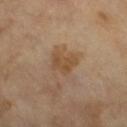follow-up — total-body-photography surveillance lesion; no biopsy
patient — male, aged 63–67
image — 15 mm crop, total-body photography
size — about 4 mm
tile lighting — cross-polarized